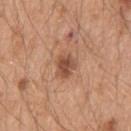The lesion was tiled from a total-body skin photograph and was not biopsied. Longest diameter approximately 3 mm. The lesion is on the left upper arm. A 15 mm crop from a total-body photograph taken for skin-cancer surveillance. A male subject, aged around 50. Captured under white-light illumination.